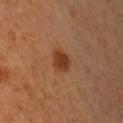Findings:
- biopsy status: no biopsy performed (imaged during a skin exam)
- automated lesion analysis: an eccentricity of roughly 0.6 and a shape-asymmetry score of about 0.2 (0 = symmetric); a lesion color around L≈38 a*≈25 b*≈34 in CIELAB and roughly 10 lightness units darker than nearby skin; a border-irregularity rating of about 1.5/10 and a within-lesion color-variation index near 2.5/10
- acquisition: ~15 mm tile from a whole-body skin photo
- subject: male, about 50 years old
- body site: the right upper arm
- lighting: cross-polarized
- size: ~3 mm (longest diameter)